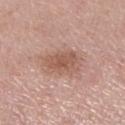The lesion was tiled from a total-body skin photograph and was not biopsied.
The tile uses white-light illumination.
From the right lower leg.
Automated image analysis of the tile measured an area of roughly 11 mm², a shape eccentricity near 0.75, and a shape-asymmetry score of about 0.25 (0 = symmetric). It also reported roughly 9 lightness units darker than nearby skin and a normalized border contrast of about 6.5. The software also gave an automated nevus-likeness rating near 20 out of 100 and a detector confidence of about 100 out of 100 that the crop contains a lesion.
The patient is a female aged 68 to 72.
The recorded lesion diameter is about 4.5 mm.
Cropped from a total-body skin-imaging series; the visible field is about 15 mm.